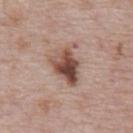Case summary:
- follow-up — imaged on a skin check; not biopsied